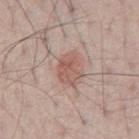Imaged during a routine full-body skin examination; the lesion was not biopsied and no histopathology is available.
Located on the abdomen.
The tile uses white-light illumination.
Cropped from a whole-body photographic skin survey; the tile spans about 15 mm.
The subject is a male in their mid-50s.
Automated tile analysis of the lesion measured a shape eccentricity near 0.7 and two-axis asymmetry of about 0.2. And it measured a lesion color around L≈56 a*≈21 b*≈25 in CIELAB and a normalized border contrast of about 6.5.
Measured at roughly 3.5 mm in maximum diameter.